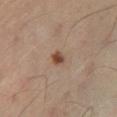Case summary:
• biopsy status: no biopsy performed (imaged during a skin exam)
• automated lesion analysis: a lesion-to-skin contrast of about 10 (normalized; higher = more distinct)
• imaging modality: 15 mm crop, total-body photography
• site: the leg
• patient: male, aged approximately 60
• lighting: cross-polarized